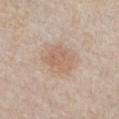No biopsy was performed on this lesion — it was imaged during a full skin examination and was not determined to be concerning. Approximately 3.5 mm at its widest. A region of skin cropped from a whole-body photographic capture, roughly 15 mm wide. The tile uses white-light illumination. On the chest. Automated tile analysis of the lesion measured border irregularity of about 2 on a 0–10 scale, a color-variation rating of about 2.5/10, and radial color variation of about 1. A male patient roughly 60 years of age.